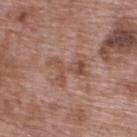{
  "automated_metrics": {
    "area_mm2_approx": 8.5,
    "shape_asymmetry": 0.45,
    "border_irregularity_0_10": 8.5,
    "color_variation_0_10": 3.5,
    "peripheral_color_asymmetry": 1.0
  },
  "image": {
    "source": "total-body photography crop",
    "field_of_view_mm": 15
  },
  "site": "upper back",
  "lesion_size": {
    "long_diameter_mm_approx": 5.0
  },
  "patient": {
    "sex": "male",
    "age_approx": 70
  },
  "lighting": "white-light"
}